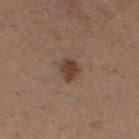The lesion was tiled from a total-body skin photograph and was not biopsied.
A 15 mm crop from a total-body photograph taken for skin-cancer surveillance.
A female patient, about 30 years old.
The lesion is on the right thigh.